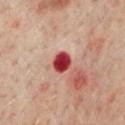Recorded during total-body skin imaging; not selected for excision or biopsy. The recorded lesion diameter is about 2.5 mm. Imaged with cross-polarized lighting. A male patient aged 63–67. A 15 mm close-up tile from a total-body photography series done for melanoma screening. On the chest. An algorithmic analysis of the crop reported an eccentricity of roughly 0.55 and a shape-asymmetry score of about 0.15 (0 = symmetric). The software also gave a lesion color around L≈41 a*≈40 b*≈26 in CIELAB and a lesion–skin lightness drop of about 21. It also reported border irregularity of about 1 on a 0–10 scale and a color-variation rating of about 2.5/10. The software also gave an automated nevus-likeness rating near 0 out of 100 and a detector confidence of about 100 out of 100 that the crop contains a lesion.